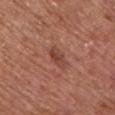Imaged during a routine full-body skin examination; the lesion was not biopsied and no histopathology is available.
This is a white-light tile.
The subject is a male roughly 75 years of age.
From the chest.
A roughly 15 mm field-of-view crop from a total-body skin photograph.